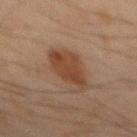notes: total-body-photography surveillance lesion; no biopsy
acquisition: total-body-photography crop, ~15 mm field of view
location: the mid back
patient: male, aged approximately 45
lesion size: ≈5.5 mm